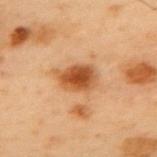biopsy status: total-body-photography surveillance lesion; no biopsy | automated metrics: an area of roughly 9 mm², an outline eccentricity of about 0.7 (0 = round, 1 = elongated), and a symmetry-axis asymmetry near 0.2; an average lesion color of about L≈43 a*≈22 b*≈35 (CIELAB), a lesion–skin lightness drop of about 13, and a normalized border contrast of about 10; lesion-presence confidence of about 100/100 | body site: the upper back | illumination: cross-polarized illumination | patient: male, aged approximately 55 | lesion diameter: ≈4 mm | acquisition: total-body-photography crop, ~15 mm field of view.